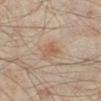  biopsy_status: not biopsied; imaged during a skin examination
  patient:
    sex: male
    age_approx: 45
  lesion_size:
    long_diameter_mm_approx: 2.5
  site: right lower leg
  image:
    source: total-body photography crop
    field_of_view_mm: 15
  lighting: cross-polarized